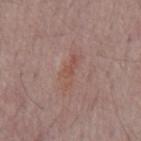biopsy status: imaged on a skin check; not biopsied.